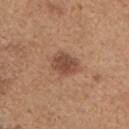| field | value |
|---|---|
| biopsy status | no biopsy performed (imaged during a skin exam) |
| automated metrics | border irregularity of about 2 on a 0–10 scale, a color-variation rating of about 2/10, and radial color variation of about 0.5; lesion-presence confidence of about 100/100 |
| imaging modality | total-body-photography crop, ~15 mm field of view |
| patient | male, about 65 years old |
| location | the chest |
| lesion size | ≈3.5 mm |